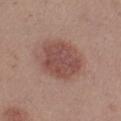{"biopsy_status": "not biopsied; imaged during a skin examination", "site": "leg", "automated_metrics": {"area_mm2_approx": 22.0, "eccentricity": 0.35, "vs_skin_darker_L": 10.0, "vs_skin_contrast_norm": 7.5, "color_variation_0_10": 3.0, "peripheral_color_asymmetry": 1.0, "nevus_likeness_0_100": 95}, "lesion_size": {"long_diameter_mm_approx": 5.5}, "patient": {"sex": "female", "age_approx": 30}, "lighting": "white-light", "image": {"source": "total-body photography crop", "field_of_view_mm": 15}}